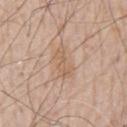This lesion was catalogued during total-body skin photography and was not selected for biopsy. This image is a 15 mm lesion crop taken from a total-body photograph. The lesion-visualizer software estimated a footprint of about 6.5 mm², an eccentricity of roughly 0.9, and a symmetry-axis asymmetry near 0.35. The analysis additionally found roughly 7 lightness units darker than nearby skin and a normalized lesion–skin contrast near 5.5. It also reported a within-lesion color-variation index near 2.5/10 and radial color variation of about 1. From the chest. A male subject, aged 78–82.